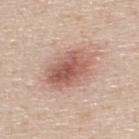Impression: The lesion was tiled from a total-body skin photograph and was not biopsied. Background: On the upper back. A roughly 15 mm field-of-view crop from a total-body skin photograph. Captured under white-light illumination. The subject is a male roughly 45 years of age. Measured at roughly 6 mm in maximum diameter.